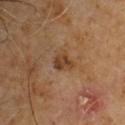The lesion was photographed on a routine skin check and not biopsied; there is no pathology result. A male subject aged around 60. The tile uses cross-polarized illumination. From the upper back. Longest diameter approximately 2.5 mm. A 15 mm close-up tile from a total-body photography series done for melanoma screening.